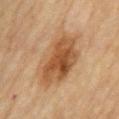<tbp_lesion>
  <biopsy_status>not biopsied; imaged during a skin examination</biopsy_status>
  <image>
    <source>total-body photography crop</source>
    <field_of_view_mm>15</field_of_view_mm>
  </image>
  <patient>
    <sex>female</sex>
    <age_approx>80</age_approx>
  </patient>
  <lesion_size>
    <long_diameter_mm_approx>8.0</long_diameter_mm_approx>
  </lesion_size>
  <lighting>cross-polarized</lighting>
  <site>mid back</site>
</tbp_lesion>